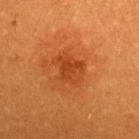| feature | finding |
|---|---|
| follow-up | total-body-photography surveillance lesion; no biopsy |
| TBP lesion metrics | a lesion area of about 9.5 mm² and two-axis asymmetry of about 0.35; a lesion-detection confidence of about 100/100 |
| subject | female, roughly 30 years of age |
| size | about 4 mm |
| lighting | cross-polarized |
| site | the upper back |
| image source | ~15 mm crop, total-body skin-cancer survey |